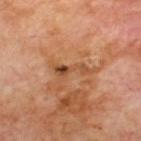Imaged during a routine full-body skin examination; the lesion was not biopsied and no histopathology is available.
Approximately 4.5 mm at its widest.
A male subject aged approximately 70.
On the upper back.
A lesion tile, about 15 mm wide, cut from a 3D total-body photograph.